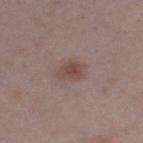Clinical impression: Imaged during a routine full-body skin examination; the lesion was not biopsied and no histopathology is available. Clinical summary: Captured under white-light illumination. The lesion is located on the leg. A 15 mm close-up tile from a total-body photography series done for melanoma screening. The patient is a female approximately 30 years of age.